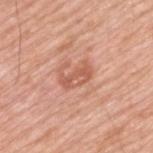Q: Is there a histopathology result?
A: catalogued during a skin exam; not biopsied